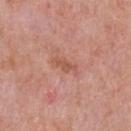Background: A male subject, aged 48 to 52. The recorded lesion diameter is about 2.5 mm. A roughly 15 mm field-of-view crop from a total-body skin photograph. The tile uses white-light illumination. From the chest.